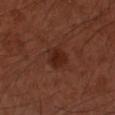This lesion was catalogued during total-body skin photography and was not selected for biopsy.